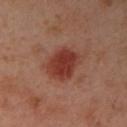The lesion was tiled from a total-body skin photograph and was not biopsied.
About 5 mm across.
The tile uses cross-polarized illumination.
Located on the left arm.
The patient is a female aged around 40.
A region of skin cropped from a whole-body photographic capture, roughly 15 mm wide.